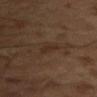Notes:
• workup — catalogued during a skin exam; not biopsied
• imaging modality — total-body-photography crop, ~15 mm field of view
• patient — male, about 65 years old